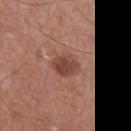follow-up = total-body-photography surveillance lesion; no biopsy
patient = male, in their mid- to late 60s
illumination = white-light
site = the left upper arm
lesion size = ~3 mm (longest diameter)
image source = ~15 mm crop, total-body skin-cancer survey
TBP lesion metrics = a lesion area of about 6 mm², a shape eccentricity near 0.6, and two-axis asymmetry of about 0.15